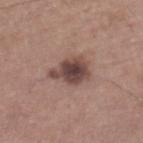notes=catalogued during a skin exam; not biopsied | location=the left lower leg | subject=male, roughly 65 years of age | size=≈4.5 mm | acquisition=~15 mm crop, total-body skin-cancer survey | tile lighting=white-light.